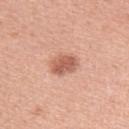This lesion was catalogued during total-body skin photography and was not selected for biopsy. On the left upper arm. This image is a 15 mm lesion crop taken from a total-body photograph. The subject is a female in their mid-40s.